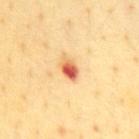Clinical summary:
From the front of the torso. A 15 mm close-up extracted from a 3D total-body photography capture. The patient is a male in their mid-60s. Measured at roughly 2.5 mm in maximum diameter. Automated tile analysis of the lesion measured an average lesion color of about L≈66 a*≈33 b*≈42 (CIELAB), about 18 CIELAB-L* units darker than the surrounding skin, and a lesion-to-skin contrast of about 10.5 (normalized; higher = more distinct). It also reported an automated nevus-likeness rating near 0 out of 100 and lesion-presence confidence of about 100/100. Captured under cross-polarized illumination.